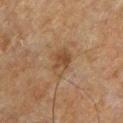The lesion was tiled from a total-body skin photograph and was not biopsied. A male subject in their 50s. A 15 mm crop from a total-body photograph taken for skin-cancer surveillance. On the chest. Longest diameter approximately 3.5 mm. The lesion-visualizer software estimated an average lesion color of about L≈37 a*≈15 b*≈27 (CIELAB) and a normalized border contrast of about 6.5. The software also gave a within-lesion color-variation index near 4/10 and radial color variation of about 1.5. It also reported lesion-presence confidence of about 100/100.